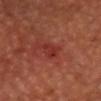- workup · no biopsy performed (imaged during a skin exam)
- patient · male, in their mid- to late 60s
- size · ~2.5 mm (longest diameter)
- TBP lesion metrics · a footprint of about 3.5 mm², a shape eccentricity near 0.75, and two-axis asymmetry of about 0.2; roughly 6 lightness units darker than nearby skin and a normalized lesion–skin contrast near 5.5
- imaging modality · 15 mm crop, total-body photography
- tile lighting · cross-polarized
- anatomic site · the head or neck